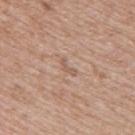This is a white-light tile. Automated tile analysis of the lesion measured an area of roughly 2 mm², an outline eccentricity of about 0.9 (0 = round, 1 = elongated), and a symmetry-axis asymmetry near 0.55. It also reported a lesion color around L≈57 a*≈18 b*≈28 in CIELAB, roughly 7 lightness units darker than nearby skin, and a normalized border contrast of about 5. And it measured a border-irregularity rating of about 6/10, a color-variation rating of about 0/10, and radial color variation of about 0. The software also gave a classifier nevus-likeness of about 0/100 and lesion-presence confidence of about 90/100. A close-up tile cropped from a whole-body skin photograph, about 15 mm across. From the upper back. Longest diameter approximately 2.5 mm. A female subject, roughly 40 years of age.